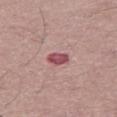Findings:
• lighting: white-light illumination
• subject: male, in their mid-50s
• lesion diameter: about 2.5 mm
• image: 15 mm crop, total-body photography
• automated lesion analysis: a border-irregularity rating of about 2/10 and peripheral color asymmetry of about 1